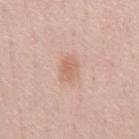{
  "biopsy_status": "not biopsied; imaged during a skin examination",
  "patient": {
    "sex": "male",
    "age_approx": 60
  },
  "site": "chest",
  "image": {
    "source": "total-body photography crop",
    "field_of_view_mm": 15
  }
}